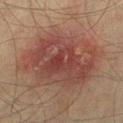| key | value |
|---|---|
| body site | the left thigh |
| lighting | cross-polarized |
| subject | male, aged around 75 |
| TBP lesion metrics | a shape-asymmetry score of about 0.15 (0 = symmetric); a mean CIELAB color near L≈37 a*≈19 b*≈22, roughly 9 lightness units darker than nearby skin, and a lesion-to-skin contrast of about 8 (normalized; higher = more distinct); a classifier nevus-likeness of about 45/100 and a lesion-detection confidence of about 100/100 |
| imaging modality | ~15 mm tile from a whole-body skin photo |
| lesion diameter | ≈10 mm |